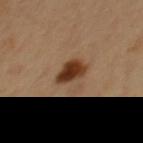<case>
<biopsy_status>not biopsied; imaged during a skin examination</biopsy_status>
<site>chest</site>
<lesion_size>
  <long_diameter_mm_approx>3.5</long_diameter_mm_approx>
</lesion_size>
<lighting>cross-polarized</lighting>
<patient>
  <sex>male</sex>
  <age_approx>50</age_approx>
</patient>
<image>
  <source>total-body photography crop</source>
  <field_of_view_mm>15</field_of_view_mm>
</image>
<automated_metrics>
  <border_irregularity_0_10>2.5</border_irregularity_0_10>
  <color_variation_0_10>4.0</color_variation_0_10>
  <nevus_likeness_0_100>100</nevus_likeness_0_100>
</automated_metrics>
</case>